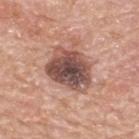Imaged during a routine full-body skin examination; the lesion was not biopsied and no histopathology is available. An algorithmic analysis of the crop reported an average lesion color of about L≈50 a*≈21 b*≈23 (CIELAB), roughly 16 lightness units darker than nearby skin, and a normalized border contrast of about 11.5. The analysis additionally found border irregularity of about 2.5 on a 0–10 scale, a within-lesion color-variation index near 8/10, and peripheral color asymmetry of about 2.5. The analysis additionally found a nevus-likeness score of about 15/100 and lesion-presence confidence of about 100/100. This is a white-light tile. Longest diameter approximately 5 mm. The lesion is located on the upper back. A close-up tile cropped from a whole-body skin photograph, about 15 mm across. A male patient aged 78 to 82.